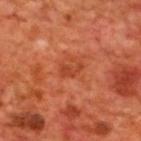Clinical impression: Part of a total-body skin-imaging series; this lesion was reviewed on a skin check and was not flagged for biopsy. Clinical summary: An algorithmic analysis of the crop reported a footprint of about 3 mm², an eccentricity of roughly 0.85, and a symmetry-axis asymmetry near 0.45. And it measured a lesion-to-skin contrast of about 6 (normalized; higher = more distinct). A close-up tile cropped from a whole-body skin photograph, about 15 mm across. The lesion is located on the upper back. A male patient, aged 68 to 72. This is a cross-polarized tile.